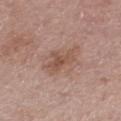No biopsy was performed on this lesion — it was imaged during a full skin examination and was not determined to be concerning.
Measured at roughly 3.5 mm in maximum diameter.
A 15 mm close-up tile from a total-body photography series done for melanoma screening.
A male subject aged 73–77.
This is a white-light tile.
The lesion is on the left thigh.